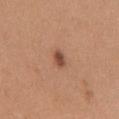<case>
  <biopsy_status>not biopsied; imaged during a skin examination</biopsy_status>
  <image>
    <source>total-body photography crop</source>
    <field_of_view_mm>15</field_of_view_mm>
  </image>
  <site>front of the torso</site>
  <patient>
    <sex>female</sex>
    <age_approx>30</age_approx>
  </patient>
</case>